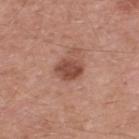Part of a total-body skin-imaging series; this lesion was reviewed on a skin check and was not flagged for biopsy. From the back. About 3 mm across. A male subject, in their mid- to late 50s. Captured under white-light illumination. A 15 mm close-up tile from a total-body photography series done for melanoma screening.